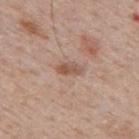follow-up=imaged on a skin check; not biopsied